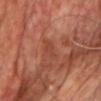The lesion was tiled from a total-body skin photograph and was not biopsied.
Cropped from a total-body skin-imaging series; the visible field is about 15 mm.
Captured under cross-polarized illumination.
The lesion's longest dimension is about 2.5 mm.
On the chest.
A male patient in their 60s.